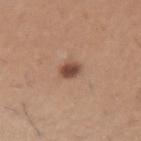The lesion was tiled from a total-body skin photograph and was not biopsied. A male patient, aged approximately 60. A 15 mm close-up tile from a total-body photography series done for melanoma screening. The tile uses white-light illumination. On the arm. Measured at roughly 2.5 mm in maximum diameter.